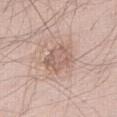notes = total-body-photography surveillance lesion; no biopsy | lesion diameter = ≈3.5 mm | lighting = white-light illumination | imaging modality = ~15 mm crop, total-body skin-cancer survey | patient = male, aged around 65 | anatomic site = the right thigh.